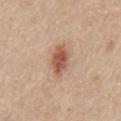Q: Is there a histopathology result?
A: imaged on a skin check; not biopsied
Q: Patient demographics?
A: male, aged approximately 65
Q: What kind of image is this?
A: 15 mm crop, total-body photography
Q: Where on the body is the lesion?
A: the chest